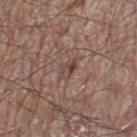Q: Was this lesion biopsied?
A: catalogued during a skin exam; not biopsied
Q: Patient demographics?
A: male, in their 60s
Q: What is the anatomic site?
A: the left thigh
Q: Lesion size?
A: about 2.5 mm
Q: What lighting was used for the tile?
A: white-light illumination
Q: What is the imaging modality?
A: total-body-photography crop, ~15 mm field of view